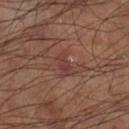image: ~15 mm tile from a whole-body skin photo; site: the right thigh; illumination: cross-polarized illumination.